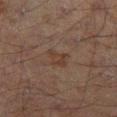The lesion was tiled from a total-body skin photograph and was not biopsied. The recorded lesion diameter is about 2.5 mm. The tile uses cross-polarized illumination. The total-body-photography lesion software estimated a mean CIELAB color near L≈30 a*≈14 b*≈22, a lesion–skin lightness drop of about 5, and a lesion-to-skin contrast of about 6 (normalized; higher = more distinct). The software also gave a nevus-likeness score of about 0/100 and a lesion-detection confidence of about 100/100. A male subject aged 68 to 72. Cropped from a total-body skin-imaging series; the visible field is about 15 mm. From the leg.